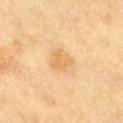notes — total-body-photography surveillance lesion; no biopsy | subject — female, aged 53–57 | illumination — cross-polarized | anatomic site — the left lower leg | image — 15 mm crop, total-body photography | image-analysis metrics — a detector confidence of about 100 out of 100 that the crop contains a lesion.